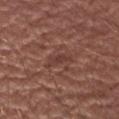| field | value |
|---|---|
| acquisition | ~15 mm tile from a whole-body skin photo |
| illumination | white-light illumination |
| body site | the right upper arm |
| image-analysis metrics | an eccentricity of roughly 0.95 and two-axis asymmetry of about 0.4; an average lesion color of about L≈38 a*≈22 b*≈24 (CIELAB), a lesion–skin lightness drop of about 7, and a lesion-to-skin contrast of about 6 (normalized; higher = more distinct); an automated nevus-likeness rating near 0 out of 100 and a detector confidence of about 65 out of 100 that the crop contains a lesion |
| subject | male, aged approximately 60 |
| size | ≈3 mm |